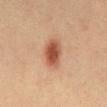This lesion was catalogued during total-body skin photography and was not selected for biopsy. A roughly 15 mm field-of-view crop from a total-body skin photograph. The subject is a male approximately 40 years of age. From the abdomen. The tile uses cross-polarized illumination.